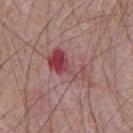Impression:
Part of a total-body skin-imaging series; this lesion was reviewed on a skin check and was not flagged for biopsy.
Clinical summary:
Automated image analysis of the tile measured a border-irregularity index near 8/10 and radial color variation of about 1.5. The lesion is on the chest. A male subject aged approximately 65. Measured at roughly 6.5 mm in maximum diameter. The tile uses white-light illumination. This image is a 15 mm lesion crop taken from a total-body photograph.